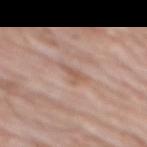  biopsy_status: not biopsied; imaged during a skin examination
  site: mid back
  patient:
    sex: male
    age_approx: 80
  image:
    source: total-body photography crop
    field_of_view_mm: 15